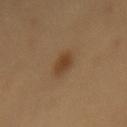location: the mid back
patient: female, about 60 years old
image source: ~15 mm tile from a whole-body skin photo
diameter: about 3 mm
illumination: cross-polarized